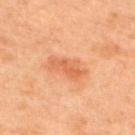workup = total-body-photography surveillance lesion; no biopsy | location = the upper back | subject = male, aged 43–47 | lighting = cross-polarized illumination | image = total-body-photography crop, ~15 mm field of view.